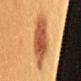  biopsy_status: not biopsied; imaged during a skin examination
  image:
    source: total-body photography crop
    field_of_view_mm: 15
  lesion_size:
    long_diameter_mm_approx: 6.5
  lighting: cross-polarized
  patient:
    sex: female
    age_approx: 40
  site: lower back